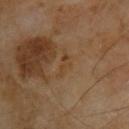workup: catalogued during a skin exam; not biopsied
lesion size: ~3 mm (longest diameter)
anatomic site: the upper back
patient: male, about 70 years old
imaging modality: ~15 mm tile from a whole-body skin photo
automated metrics: border irregularity of about 5 on a 0–10 scale, a within-lesion color-variation index near 0/10, and peripheral color asymmetry of about 0
tile lighting: cross-polarized